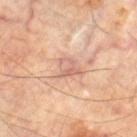Impression: No biopsy was performed on this lesion — it was imaged during a full skin examination and was not determined to be concerning. Image and clinical context: A male patient, in their 70s. The lesion is located on the left thigh. The lesion-visualizer software estimated an area of roughly 4 mm², a shape eccentricity near 0.75, and a shape-asymmetry score of about 0.35 (0 = symmetric). It also reported a mean CIELAB color near L≈63 a*≈21 b*≈28, about 8 CIELAB-L* units darker than the surrounding skin, and a lesion-to-skin contrast of about 5.5 (normalized; higher = more distinct). And it measured a border-irregularity index near 3.5/10, a within-lesion color-variation index near 4.5/10, and radial color variation of about 1.5. The software also gave a nevus-likeness score of about 0/100. Imaged with cross-polarized lighting. Measured at roughly 2.5 mm in maximum diameter. A region of skin cropped from a whole-body photographic capture, roughly 15 mm wide.